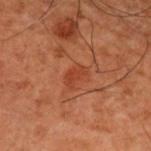Impression: The lesion was photographed on a routine skin check and not biopsied; there is no pathology result. Background: A male subject in their 50s. The lesion is located on the upper back. Imaged with cross-polarized lighting. The lesion-visualizer software estimated two-axis asymmetry of about 0.4. The software also gave a mean CIELAB color near L≈32 a*≈25 b*≈29, roughly 6 lightness units darker than nearby skin, and a normalized border contrast of about 5.5. This image is a 15 mm lesion crop taken from a total-body photograph.